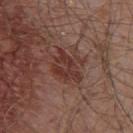Q: Was this lesion biopsied?
A: no biopsy performed (imaged during a skin exam)
Q: What lighting was used for the tile?
A: white-light illumination
Q: What is the lesion's diameter?
A: about 4 mm
Q: Patient demographics?
A: male, aged around 55
Q: What kind of image is this?
A: ~15 mm tile from a whole-body skin photo
Q: Lesion location?
A: the front of the torso